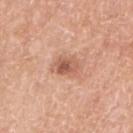* follow-up · imaged on a skin check; not biopsied
* imaging modality · ~15 mm tile from a whole-body skin photo
* subject · female, roughly 75 years of age
* body site · the right upper arm